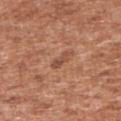<tbp_lesion>
<biopsy_status>not biopsied; imaged during a skin examination</biopsy_status>
<site>right upper arm</site>
<lighting>white-light</lighting>
<image>
  <source>total-body photography crop</source>
  <field_of_view_mm>15</field_of_view_mm>
</image>
<patient>
  <sex>male</sex>
  <age_approx>45</age_approx>
</patient>
<lesion_size>
  <long_diameter_mm_approx>3.0</long_diameter_mm_approx>
</lesion_size>
</tbp_lesion>